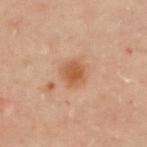Case summary:
* imaging modality — total-body-photography crop, ~15 mm field of view
* site — the back
* tile lighting — cross-polarized
* lesion size — ~3 mm (longest diameter)
* patient — female, in their 40s